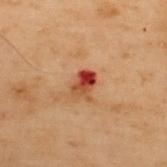follow-up: total-body-photography surveillance lesion; no biopsy
anatomic site: the upper back
acquisition: total-body-photography crop, ~15 mm field of view
automated lesion analysis: an average lesion color of about L≈37 a*≈27 b*≈30 (CIELAB)
diameter: ~3 mm (longest diameter)
illumination: cross-polarized illumination
patient: male, in their mid- to late 50s